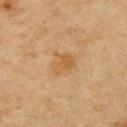follow-up = catalogued during a skin exam; not biopsied
illumination = cross-polarized
patient = female, aged 58 to 62
lesion diameter = ~3 mm (longest diameter)
image source = ~15 mm crop, total-body skin-cancer survey
TBP lesion metrics = a footprint of about 7 mm² and an eccentricity of roughly 0.4; about 6 CIELAB-L* units darker than the surrounding skin
location = the arm